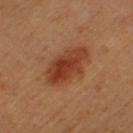Assessment: Recorded during total-body skin imaging; not selected for excision or biopsy. Background: The recorded lesion diameter is about 6 mm. Cropped from a total-body skin-imaging series; the visible field is about 15 mm. Automated image analysis of the tile measured a footprint of about 14 mm². The subject is a female aged approximately 30. Located on the left thigh.